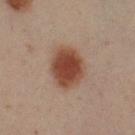follow-up: total-body-photography surveillance lesion; no biopsy | anatomic site: the left forearm | size: ~5 mm (longest diameter) | subject: male, aged approximately 50 | illumination: cross-polarized | image source: total-body-photography crop, ~15 mm field of view.